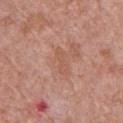Background: The tile uses white-light illumination. From the chest. A roughly 15 mm field-of-view crop from a total-body skin photograph. Measured at roughly 3.5 mm in maximum diameter. A male patient approximately 50 years of age.